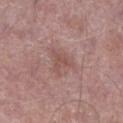Captured during whole-body skin photography for melanoma surveillance; the lesion was not biopsied. A region of skin cropped from a whole-body photographic capture, roughly 15 mm wide. The lesion is on the leg. Imaged with white-light lighting. The patient is a male approximately 55 years of age. Automated image analysis of the tile measured a mean CIELAB color near L≈52 a*≈21 b*≈23 and a lesion-to-skin contrast of about 5.5 (normalized; higher = more distinct). The software also gave a border-irregularity index near 6.5/10 and radial color variation of about 0.5. It also reported a classifier nevus-likeness of about 0/100 and a lesion-detection confidence of about 100/100. About 3 mm across.